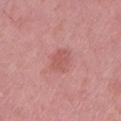Automated image analysis of the tile measured an eccentricity of roughly 0.65 and two-axis asymmetry of about 0.25. The analysis additionally found an average lesion color of about L≈56 a*≈28 b*≈25 (CIELAB), about 7 CIELAB-L* units darker than the surrounding skin, and a lesion-to-skin contrast of about 5.5 (normalized; higher = more distinct). It also reported a border-irregularity index near 2/10, a color-variation rating of about 1.5/10, and peripheral color asymmetry of about 0.5. The analysis additionally found a classifier nevus-likeness of about 10/100 and lesion-presence confidence of about 100/100.
Located on the left lower leg.
A female subject, in their 40s.
Cropped from a total-body skin-imaging series; the visible field is about 15 mm.
The lesion's longest dimension is about 3 mm.
Imaged with white-light lighting.